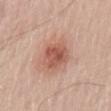follow-up = total-body-photography surveillance lesion; no biopsy | automated lesion analysis = an area of roughly 9.5 mm², an eccentricity of roughly 0.6, and two-axis asymmetry of about 0.2; a lesion color around L≈55 a*≈25 b*≈29 in CIELAB | location = the back | lighting = white-light illumination | lesion diameter = ~4 mm (longest diameter) | patient = male, aged approximately 70 | acquisition = ~15 mm crop, total-body skin-cancer survey.